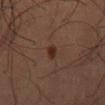Impression: Recorded during total-body skin imaging; not selected for excision or biopsy. Background: A region of skin cropped from a whole-body photographic capture, roughly 15 mm wide. From the back. An algorithmic analysis of the crop reported a mean CIELAB color near L≈28 a*≈18 b*≈23, a lesion–skin lightness drop of about 8, and a lesion-to-skin contrast of about 9 (normalized; higher = more distinct). It also reported an automated nevus-likeness rating near 85 out of 100 and a lesion-detection confidence of about 100/100. Measured at roughly 2.5 mm in maximum diameter. A male subject, in their mid- to late 60s.